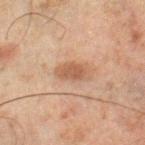Q: How was this image acquired?
A: total-body-photography crop, ~15 mm field of view
Q: What did automated image analysis measure?
A: a color-variation rating of about 1.5/10 and peripheral color asymmetry of about 0.5
Q: How was the tile lit?
A: cross-polarized illumination
Q: How large is the lesion?
A: ~3.5 mm (longest diameter)
Q: Patient demographics?
A: male, roughly 45 years of age
Q: Lesion location?
A: the right lower leg